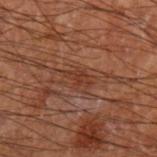Assessment:
The lesion was photographed on a routine skin check and not biopsied; there is no pathology result.
Clinical summary:
A male subject, about 70 years old. Located on the left forearm. Approximately 3 mm at its widest. A region of skin cropped from a whole-body photographic capture, roughly 15 mm wide.